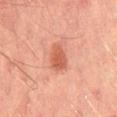Assessment: The lesion was photographed on a routine skin check and not biopsied; there is no pathology result. Clinical summary: Located on the mid back. The recorded lesion diameter is about 3.5 mm. This image is a 15 mm lesion crop taken from a total-body photograph. A male patient, in their mid-40s.